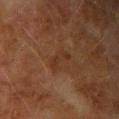The lesion was photographed on a routine skin check and not biopsied; there is no pathology result.
Automated tile analysis of the lesion measured a lesion area of about 4 mm², a shape eccentricity near 0.9, and a symmetry-axis asymmetry near 0.5. The analysis additionally found a nevus-likeness score of about 0/100 and lesion-presence confidence of about 100/100.
A lesion tile, about 15 mm wide, cut from a 3D total-body photograph.
A male patient in their mid- to late 70s.
Located on the right upper arm.
Captured under cross-polarized illumination.